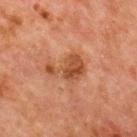workup — imaged on a skin check; not biopsied
anatomic site — the chest
patient — male, about 65 years old
acquisition — total-body-photography crop, ~15 mm field of view